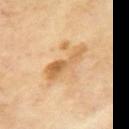Imaged during a routine full-body skin examination; the lesion was not biopsied and no histopathology is available.
The tile uses cross-polarized illumination.
A male subject, aged approximately 70.
A 15 mm close-up tile from a total-body photography series done for melanoma screening.
From the left upper arm.
The lesion-visualizer software estimated an area of roughly 9 mm², an eccentricity of roughly 0.95, and a shape-asymmetry score of about 0.4 (0 = symmetric). It also reported about 10 CIELAB-L* units darker than the surrounding skin and a normalized border contrast of about 7. The software also gave a nevus-likeness score of about 0/100.